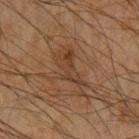Impression: Captured during whole-body skin photography for melanoma surveillance; the lesion was not biopsied.